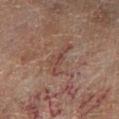biopsy status: no biopsy performed (imaged during a skin exam); site: the left lower leg; image: total-body-photography crop, ~15 mm field of view; patient: male, about 60 years old.